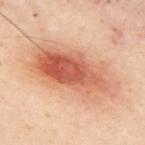Q: Was this lesion biopsied?
A: imaged on a skin check; not biopsied
Q: Where on the body is the lesion?
A: the upper back
Q: What is the lesion's diameter?
A: ~10 mm (longest diameter)
Q: What did automated image analysis measure?
A: a footprint of about 43 mm², an outline eccentricity of about 0.85 (0 = round, 1 = elongated), and a symmetry-axis asymmetry near 0.1; a mean CIELAB color near L≈51 a*≈21 b*≈29 and about 11 CIELAB-L* units darker than the surrounding skin; a nevus-likeness score of about 100/100 and lesion-presence confidence of about 100/100
Q: Illumination type?
A: cross-polarized illumination
Q: Who is the patient?
A: male, aged 48 to 52
Q: How was this image acquired?
A: ~15 mm crop, total-body skin-cancer survey